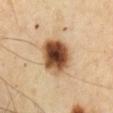Q: Was this lesion biopsied?
A: total-body-photography surveillance lesion; no biopsy
Q: How was this image acquired?
A: ~15 mm tile from a whole-body skin photo
Q: Patient demographics?
A: male, about 65 years old
Q: What is the lesion's diameter?
A: about 5 mm
Q: Where on the body is the lesion?
A: the right upper arm
Q: Illumination type?
A: cross-polarized